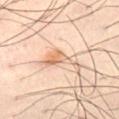This lesion was catalogued during total-body skin photography and was not selected for biopsy.
Captured under cross-polarized illumination.
A male subject aged approximately 40.
Approximately 5.5 mm at its widest.
A roughly 15 mm field-of-view crop from a total-body skin photograph.
From the left thigh.